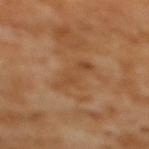Imaged during a routine full-body skin examination; the lesion was not biopsied and no histopathology is available. The subject is a female roughly 55 years of age. Cropped from a total-body skin-imaging series; the visible field is about 15 mm. The lesion is on the mid back.